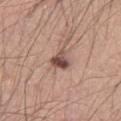The lesion was tiled from a total-body skin photograph and was not biopsied.
Measured at roughly 3 mm in maximum diameter.
Cropped from a total-body skin-imaging series; the visible field is about 15 mm.
The lesion is on the left thigh.
A male patient, approximately 40 years of age.
This is a white-light tile.